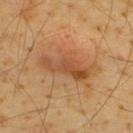Clinical impression:
Recorded during total-body skin imaging; not selected for excision or biopsy.
Acquisition and patient details:
A 15 mm crop from a total-body photograph taken for skin-cancer surveillance. The total-body-photography lesion software estimated an area of roughly 12 mm². The software also gave a classifier nevus-likeness of about 95/100 and a lesion-detection confidence of about 100/100. On the upper back. A male subject approximately 65 years of age. Imaged with cross-polarized lighting.